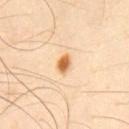Q: Is there a histopathology result?
A: total-body-photography surveillance lesion; no biopsy
Q: What lighting was used for the tile?
A: cross-polarized
Q: What is the lesion's diameter?
A: ≈2.5 mm
Q: Lesion location?
A: the chest
Q: Patient demographics?
A: male, about 45 years old
Q: What did automated image analysis measure?
A: a shape eccentricity near 0.7 and a shape-asymmetry score of about 0.2 (0 = symmetric); a mean CIELAB color near L≈66 a*≈24 b*≈44
Q: What is the imaging modality?
A: ~15 mm crop, total-body skin-cancer survey